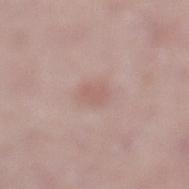biopsy status — total-body-photography surveillance lesion; no biopsy
patient — male, aged approximately 50
lighting — white-light
lesion diameter — ≈2.5 mm
image — ~15 mm crop, total-body skin-cancer survey
location — the left lower leg